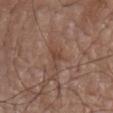Findings:
• illumination: white-light illumination
• subject: male, aged 73–77
• anatomic site: the chest
• imaging modality: total-body-photography crop, ~15 mm field of view
• TBP lesion metrics: a classifier nevus-likeness of about 0/100 and lesion-presence confidence of about 95/100